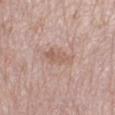Captured during whole-body skin photography for melanoma surveillance; the lesion was not biopsied.